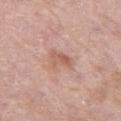Findings:
* image source: total-body-photography crop, ~15 mm field of view
* anatomic site: the right thigh
* patient: female, aged 68 to 72